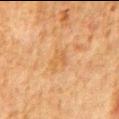Impression: Recorded during total-body skin imaging; not selected for excision or biopsy. Acquisition and patient details: This is a cross-polarized tile. The lesion is located on the abdomen. An algorithmic analysis of the crop reported a footprint of about 4.5 mm², an outline eccentricity of about 0.3 (0 = round, 1 = elongated), and two-axis asymmetry of about 0.4. It also reported a border-irregularity index near 4.5/10 and radial color variation of about 0.5. And it measured a detector confidence of about 100 out of 100 that the crop contains a lesion. A male patient roughly 80 years of age. A roughly 15 mm field-of-view crop from a total-body skin photograph. The lesion's longest dimension is about 2.5 mm.